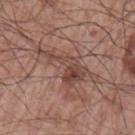This lesion was catalogued during total-body skin photography and was not selected for biopsy.
A lesion tile, about 15 mm wide, cut from a 3D total-body photograph.
The lesion is located on the left upper arm.
The patient is a male aged 63 to 67.
This is a white-light tile.